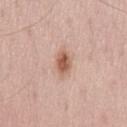Part of a total-body skin-imaging series; this lesion was reviewed on a skin check and was not flagged for biopsy.
Longest diameter approximately 3.5 mm.
A male patient, aged approximately 60.
This image is a 15 mm lesion crop taken from a total-body photograph.
Imaged with white-light lighting.
Located on the back.